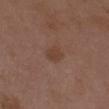Clinical summary: A female subject in their mid- to late 30s. A close-up tile cropped from a whole-body skin photograph, about 15 mm across. Located on the chest.